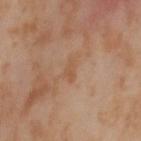Imaged during a routine full-body skin examination; the lesion was not biopsied and no histopathology is available.
Cropped from a total-body skin-imaging series; the visible field is about 15 mm.
A female patient, aged 53–57.
On the left thigh.